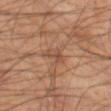{
  "biopsy_status": "not biopsied; imaged during a skin examination",
  "patient": {
    "sex": "male",
    "age_approx": 55
  },
  "image": {
    "source": "total-body photography crop",
    "field_of_view_mm": 15
  },
  "site": "left thigh"
}